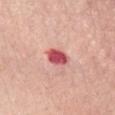The lesion was photographed on a routine skin check and not biopsied; there is no pathology result. The subject is a female about 65 years old. A 15 mm close-up tile from a total-body photography series done for melanoma screening. From the abdomen.